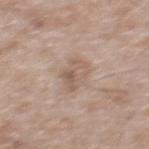Imaged during a routine full-body skin examination; the lesion was not biopsied and no histopathology is available.